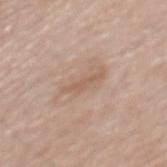The lesion was photographed on a routine skin check and not biopsied; there is no pathology result.
The patient is a male aged 53 to 57.
Imaged with white-light lighting.
The lesion is on the mid back.
A 15 mm close-up extracted from a 3D total-body photography capture.
About 4 mm across.